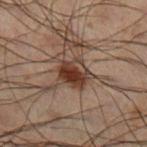No biopsy was performed on this lesion — it was imaged during a full skin examination and was not determined to be concerning. About 3.5 mm across. A male subject, aged 48–52. Captured under cross-polarized illumination. A 15 mm crop from a total-body photograph taken for skin-cancer surveillance. Located on the right lower leg.